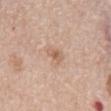Assessment:
Captured during whole-body skin photography for melanoma surveillance; the lesion was not biopsied.
Context:
A male patient, in their mid-60s. A 15 mm close-up extracted from a 3D total-body photography capture. Located on the back.